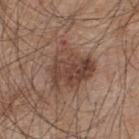The lesion was photographed on a routine skin check and not biopsied; there is no pathology result. Cropped from a total-body skin-imaging series; the visible field is about 15 mm. The tile uses white-light illumination. The subject is a male approximately 45 years of age. The lesion-visualizer software estimated a mean CIELAB color near L≈43 a*≈18 b*≈25, about 10 CIELAB-L* units darker than the surrounding skin, and a normalized border contrast of about 8. And it measured a border-irregularity rating of about 4.5/10, a within-lesion color-variation index near 5.5/10, and a peripheral color-asymmetry measure near 2. And it measured an automated nevus-likeness rating near 20 out of 100 and lesion-presence confidence of about 100/100. The lesion is located on the upper back. The recorded lesion diameter is about 5.5 mm.